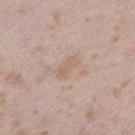Imaged during a routine full-body skin examination; the lesion was not biopsied and no histopathology is available. A 15 mm crop from a total-body photograph taken for skin-cancer surveillance. On the leg. A female patient about 25 years old.